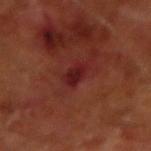A region of skin cropped from a whole-body photographic capture, roughly 15 mm wide. A male subject in their mid-60s. The lesion is on the right forearm. Imaged with cross-polarized lighting.